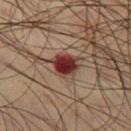Impression: No biopsy was performed on this lesion — it was imaged during a full skin examination and was not determined to be concerning. Context: The patient is a male aged approximately 50. Cropped from a total-body skin-imaging series; the visible field is about 15 mm. The lesion is on the leg.